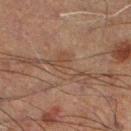Case summary:
* notes — total-body-photography surveillance lesion; no biopsy
* anatomic site — the left lower leg
* subject — male, roughly 60 years of age
* lesion size — about 4.5 mm
* image-analysis metrics — a lesion area of about 5.5 mm² and two-axis asymmetry of about 0.65; a border-irregularity rating of about 8.5/10, internal color variation of about 1 on a 0–10 scale, and a peripheral color-asymmetry measure near 0.5; a detector confidence of about 90 out of 100 that the crop contains a lesion
* acquisition — 15 mm crop, total-body photography
* lighting — cross-polarized illumination